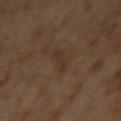{"biopsy_status": "not biopsied; imaged during a skin examination", "lighting": "cross-polarized", "automated_metrics": {"vs_skin_darker_L": 4.0, "vs_skin_contrast_norm": 5.0, "border_irregularity_0_10": 5.0, "color_variation_0_10": 0.0, "nevus_likeness_0_100": 0, "lesion_detection_confidence_0_100": 95}, "patient": {"sex": "male", "age_approx": 60}, "lesion_size": {"long_diameter_mm_approx": 4.0}, "site": "back", "image": {"source": "total-body photography crop", "field_of_view_mm": 15}}